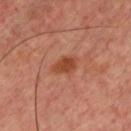Acquisition and patient details:
A region of skin cropped from a whole-body photographic capture, roughly 15 mm wide. The lesion-visualizer software estimated an average lesion color of about L≈43 a*≈26 b*≈32 (CIELAB), about 9 CIELAB-L* units darker than the surrounding skin, and a normalized border contrast of about 8. The software also gave an automated nevus-likeness rating near 75 out of 100 and lesion-presence confidence of about 100/100. Captured under cross-polarized illumination. From the chest. The lesion's longest dimension is about 3 mm. The subject is a male aged 43–47.